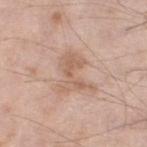Impression: The lesion was photographed on a routine skin check and not biopsied; there is no pathology result. Acquisition and patient details: A male subject aged 58–62. The lesion's longest dimension is about 4.5 mm. The lesion is located on the left lower leg. A 15 mm close-up extracted from a 3D total-body photography capture. Captured under white-light illumination. An algorithmic analysis of the crop reported a lesion color around L≈61 a*≈18 b*≈29 in CIELAB, roughly 8 lightness units darker than nearby skin, and a normalized border contrast of about 5.5. And it measured border irregularity of about 6 on a 0–10 scale, a within-lesion color-variation index near 2.5/10, and radial color variation of about 1.